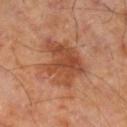Acquisition and patient details: Captured under cross-polarized illumination. A lesion tile, about 15 mm wide, cut from a 3D total-body photograph. The subject is a male about 70 years old. On the right lower leg. Longest diameter approximately 6 mm.